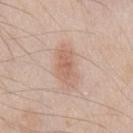imaging modality=15 mm crop, total-body photography; location=the chest; patient=male, in their mid-40s.